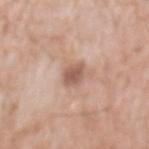| key | value |
|---|---|
| notes | no biopsy performed (imaged during a skin exam) |
| anatomic site | the mid back |
| acquisition | 15 mm crop, total-body photography |
| illumination | white-light illumination |
| patient | male, aged 53–57 |